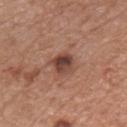workup: total-body-photography surveillance lesion; no biopsy
anatomic site: the chest
illumination: white-light illumination
patient: male, in their mid-70s
acquisition: ~15 mm crop, total-body skin-cancer survey
diameter: about 3 mm